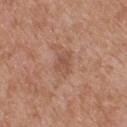Captured during whole-body skin photography for melanoma surveillance; the lesion was not biopsied. From the upper back. Imaged with white-light lighting. A region of skin cropped from a whole-body photographic capture, roughly 15 mm wide. The patient is a male aged 58–62. About 3 mm across.